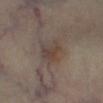biopsy status: no biopsy performed (imaged during a skin exam) | subject: male, about 70 years old | anatomic site: the leg | acquisition: total-body-photography crop, ~15 mm field of view.